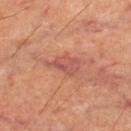Q: Was a biopsy performed?
A: no biopsy performed (imaged during a skin exam)
Q: Where on the body is the lesion?
A: the left thigh
Q: Lesion size?
A: ≈4 mm
Q: What kind of image is this?
A: ~15 mm crop, total-body skin-cancer survey
Q: What are the patient's age and sex?
A: male, aged 58–62